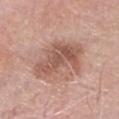Q: How large is the lesion?
A: ≈5.5 mm
Q: Patient demographics?
A: male, aged 68–72
Q: What did automated image analysis measure?
A: a lesion area of about 18 mm² and a symmetry-axis asymmetry near 0.35; roughly 11 lightness units darker than nearby skin and a normalized lesion–skin contrast near 7.5
Q: What lighting was used for the tile?
A: white-light
Q: What kind of image is this?
A: ~15 mm tile from a whole-body skin photo
Q: Where on the body is the lesion?
A: the head or neck